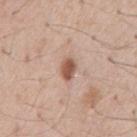The lesion was tiled from a total-body skin photograph and was not biopsied. The lesion-visualizer software estimated a lesion area of about 4 mm², an eccentricity of roughly 0.75, and a symmetry-axis asymmetry near 0.2. It also reported an average lesion color of about L≈55 a*≈21 b*≈28 (CIELAB) and a normalized lesion–skin contrast near 9.5. And it measured a border-irregularity index near 1.5/10. Cropped from a whole-body photographic skin survey; the tile spans about 15 mm. Located on the abdomen. A male subject in their mid- to late 50s. The tile uses white-light illumination.